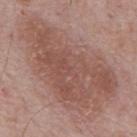Notes:
– follow-up · catalogued during a skin exam; not biopsied
– automated metrics · an average lesion color of about L≈53 a*≈20 b*≈24 (CIELAB), a lesion–skin lightness drop of about 8, and a lesion-to-skin contrast of about 6 (normalized; higher = more distinct); lesion-presence confidence of about 100/100
– diameter · ≈14.5 mm
– site · the mid back
– subject · male, in their mid-60s
– acquisition · 15 mm crop, total-body photography